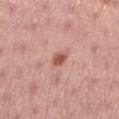workup — total-body-photography surveillance lesion; no biopsy
site — the left lower leg
illumination — white-light illumination
diameter — ~2.5 mm (longest diameter)
imaging modality — 15 mm crop, total-body photography
automated lesion analysis — an area of roughly 3.5 mm², a shape eccentricity near 0.75, and a shape-asymmetry score of about 0.2 (0 = symmetric); border irregularity of about 2 on a 0–10 scale, a color-variation rating of about 1.5/10, and a peripheral color-asymmetry measure near 0.5
patient — female, about 25 years old